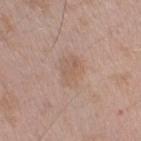workup: no biopsy performed (imaged during a skin exam) | subject: male, roughly 50 years of age | diameter: about 3.5 mm | imaging modality: ~15 mm tile from a whole-body skin photo | TBP lesion metrics: a lesion color around L≈58 a*≈17 b*≈27 in CIELAB, about 6 CIELAB-L* units darker than the surrounding skin, and a normalized border contrast of about 4.5; border irregularity of about 3 on a 0–10 scale, internal color variation of about 3 on a 0–10 scale, and peripheral color asymmetry of about 1 | anatomic site: the right upper arm.